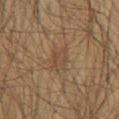biopsy status=total-body-photography surveillance lesion; no biopsy
image source=total-body-photography crop, ~15 mm field of view
diameter=≈3 mm
subject=male, aged 58 to 62
anatomic site=the mid back
lighting=cross-polarized illumination